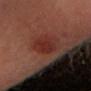| feature | finding |
|---|---|
| workup | no biopsy performed (imaged during a skin exam) |
| site | the head or neck |
| patient | male, in their 50s |
| imaging modality | total-body-photography crop, ~15 mm field of view |
| illumination | cross-polarized illumination |
| lesion size | ~5.5 mm (longest diameter) |